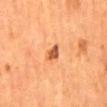| feature | finding |
|---|---|
| notes | no biopsy performed (imaged during a skin exam) |
| anatomic site | the mid back |
| lighting | cross-polarized |
| acquisition | total-body-photography crop, ~15 mm field of view |
| patient | male, aged 53 to 57 |
| lesion diameter | ≈3 mm |